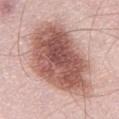location = the left thigh | lesion size = about 10.5 mm | subject = male, in their mid- to late 50s | imaging modality = 15 mm crop, total-body photography | tile lighting = white-light illumination.